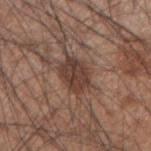workup: no biopsy performed (imaged during a skin exam).